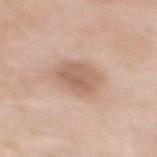Notes:
• follow-up · total-body-photography surveillance lesion; no biopsy
• illumination · white-light
• image-analysis metrics · a mean CIELAB color near L≈62 a*≈18 b*≈29, about 10 CIELAB-L* units darker than the surrounding skin, and a normalized lesion–skin contrast near 6.5
• lesion size · about 5 mm
• location · the upper back
• imaging modality · ~15 mm crop, total-body skin-cancer survey
• subject · female, aged 48 to 52